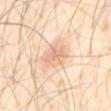The lesion was tiled from a total-body skin photograph and was not biopsied. A male subject aged 38 to 42. On the mid back. The tile uses cross-polarized illumination. Automated image analysis of the tile measured an area of roughly 8.5 mm² and a shape eccentricity near 0.6. The analysis additionally found a detector confidence of about 100 out of 100 that the crop contains a lesion. A 15 mm close-up tile from a total-body photography series done for melanoma screening. The recorded lesion diameter is about 3.5 mm.